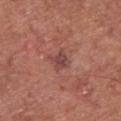* body site · the front of the torso
* image source · ~15 mm crop, total-body skin-cancer survey
* TBP lesion metrics · a shape eccentricity near 0.55; a lesion color around L≈45 a*≈24 b*≈23 in CIELAB, about 9 CIELAB-L* units darker than the surrounding skin, and a normalized border contrast of about 7; a border-irregularity index near 2/10 and a peripheral color-asymmetry measure near 0.5; lesion-presence confidence of about 100/100
* patient · male, roughly 75 years of age